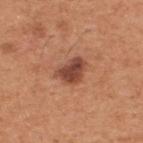workup: no biopsy performed (imaged during a skin exam); tile lighting: white-light; image source: ~15 mm crop, total-body skin-cancer survey; patient: male, in their mid-50s; lesion diameter: ~4 mm (longest diameter); location: the upper back.